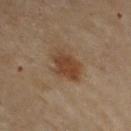A close-up tile cropped from a whole-body skin photograph, about 15 mm across.
Imaged with cross-polarized lighting.
A female patient about 60 years old.
Located on the right upper arm.
Approximately 5 mm at its widest.